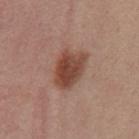Part of a total-body skin-imaging series; this lesion was reviewed on a skin check and was not flagged for biopsy. Imaged with white-light lighting. A female subject, in their mid- to late 50s. The lesion is located on the mid back. This image is a 15 mm lesion crop taken from a total-body photograph.